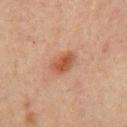A male subject in their mid- to late 60s. This is a cross-polarized tile. A 15 mm crop from a total-body photograph taken for skin-cancer surveillance. On the mid back.